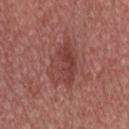Recorded during total-body skin imaging; not selected for excision or biopsy.
This is a white-light tile.
A male patient, roughly 30 years of age.
This image is a 15 mm lesion crop taken from a total-body photograph.
The recorded lesion diameter is about 6 mm.
The lesion-visualizer software estimated a footprint of about 14 mm² and two-axis asymmetry of about 0.25. And it measured a lesion-to-skin contrast of about 6 (normalized; higher = more distinct).